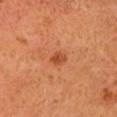Impression: This lesion was catalogued during total-body skin photography and was not selected for biopsy. Background: On the head or neck. A male patient roughly 55 years of age. Automated tile analysis of the lesion measured a lesion color around L≈50 a*≈32 b*≈42 in CIELAB, roughly 10 lightness units darker than nearby skin, and a normalized lesion–skin contrast near 7.5. The software also gave border irregularity of about 3 on a 0–10 scale, a color-variation rating of about 2.5/10, and peripheral color asymmetry of about 1. The analysis additionally found an automated nevus-likeness rating near 85 out of 100 and lesion-presence confidence of about 100/100. A roughly 15 mm field-of-view crop from a total-body skin photograph.